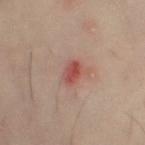Image and clinical context: On the left thigh. A 15 mm close-up tile from a total-body photography series done for melanoma screening. A female patient, aged 53 to 57.